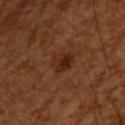Assessment: No biopsy was performed on this lesion — it was imaged during a full skin examination and was not determined to be concerning. Background: This is a cross-polarized tile. The patient is a male aged 63 to 67. The total-body-photography lesion software estimated a mean CIELAB color near L≈20 a*≈18 b*≈24 and a lesion–skin lightness drop of about 6. And it measured a color-variation rating of about 4/10. It also reported an automated nevus-likeness rating near 65 out of 100 and lesion-presence confidence of about 100/100. About 2.5 mm across. A 15 mm close-up tile from a total-body photography series done for melanoma screening. On the upper back.